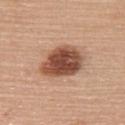{
  "lighting": "white-light",
  "image": {
    "source": "total-body photography crop",
    "field_of_view_mm": 15
  },
  "lesion_size": {
    "long_diameter_mm_approx": 6.0
  },
  "site": "upper back",
  "patient": {
    "sex": "female",
    "age_approx": 65
  }
}